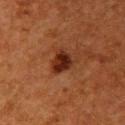Impression:
Part of a total-body skin-imaging series; this lesion was reviewed on a skin check and was not flagged for biopsy.
Acquisition and patient details:
A female patient, roughly 50 years of age. This image is a 15 mm lesion crop taken from a total-body photograph. This is a cross-polarized tile. Measured at roughly 3 mm in maximum diameter. The lesion is on the right upper arm.